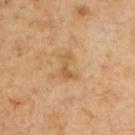{"automated_metrics": {"area_mm2_approx": 3.5, "eccentricity": 0.9, "shape_asymmetry": 0.6, "cielab_L": 56, "cielab_a": 19, "cielab_b": 39, "vs_skin_darker_L": 8.0}, "patient": {"sex": "male", "age_approx": 65}, "lighting": "cross-polarized", "lesion_size": {"long_diameter_mm_approx": 3.0}, "image": {"source": "total-body photography crop", "field_of_view_mm": 15}}